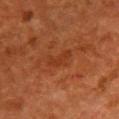Notes:
– notes — imaged on a skin check; not biopsied
– patient — female, approximately 50 years of age
– anatomic site — the front of the torso
– illumination — cross-polarized illumination
– image source — ~15 mm crop, total-body skin-cancer survey
– lesion diameter — ~3.5 mm (longest diameter)